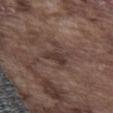biopsy status: catalogued during a skin exam; not biopsied | imaging modality: 15 mm crop, total-body photography | subject: male, roughly 75 years of age | lighting: white-light | diameter: ~3.5 mm (longest diameter) | site: the abdomen.